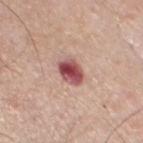Q: Was this lesion biopsied?
A: imaged on a skin check; not biopsied
Q: Who is the patient?
A: male, about 65 years old
Q: What is the anatomic site?
A: the chest
Q: What is the lesion's diameter?
A: about 3 mm
Q: What is the imaging modality?
A: ~15 mm tile from a whole-body skin photo
Q: What did automated image analysis measure?
A: an area of roughly 6.5 mm², a shape eccentricity near 0.6, and two-axis asymmetry of about 0.2; about 18 CIELAB-L* units darker than the surrounding skin and a normalized lesion–skin contrast near 12; a lesion-detection confidence of about 100/100
Q: What lighting was used for the tile?
A: white-light illumination